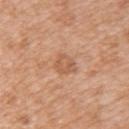{
  "biopsy_status": "not biopsied; imaged during a skin examination",
  "automated_metrics": {
    "area_mm2_approx": 5.5,
    "eccentricity": 0.5,
    "shape_asymmetry": 0.2,
    "cielab_L": 58,
    "cielab_a": 23,
    "cielab_b": 34,
    "vs_skin_darker_L": 8.0,
    "vs_skin_contrast_norm": 5.5
  },
  "lesion_size": {
    "long_diameter_mm_approx": 2.5
  },
  "image": {
    "source": "total-body photography crop",
    "field_of_view_mm": 15
  },
  "lighting": "white-light",
  "patient": {
    "sex": "female",
    "age_approx": 40
  },
  "site": "back"
}